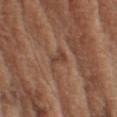The lesion is on the left upper arm. A male patient, about 75 years old. The lesion-visualizer software estimated an area of roughly 3 mm², an eccentricity of roughly 0.9, and a shape-asymmetry score of about 0.5 (0 = symmetric). Cropped from a total-body skin-imaging series; the visible field is about 15 mm. About 2.5 mm across.